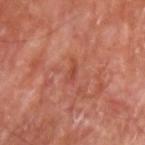Findings:
- biopsy status: total-body-photography surveillance lesion; no biopsy
- imaging modality: total-body-photography crop, ~15 mm field of view
- image-analysis metrics: border irregularity of about 4.5 on a 0–10 scale, internal color variation of about 0 on a 0–10 scale, and a peripheral color-asymmetry measure near 0
- tile lighting: cross-polarized illumination
- lesion diameter: about 2.5 mm
- patient: male, approximately 70 years of age
- site: the arm